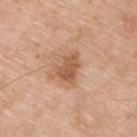Captured during whole-body skin photography for melanoma surveillance; the lesion was not biopsied. The subject is a male aged approximately 75. The tile uses white-light illumination. The lesion's longest dimension is about 3.5 mm. The lesion is on the upper back. Cropped from a whole-body photographic skin survey; the tile spans about 15 mm.